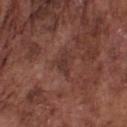The lesion was tiled from a total-body skin photograph and was not biopsied. A male patient, aged approximately 75. Located on the chest. Cropped from a whole-body photographic skin survey; the tile spans about 15 mm. Captured under white-light illumination. The total-body-photography lesion software estimated a footprint of about 3 mm², an outline eccentricity of about 0.85 (0 = round, 1 = elongated), and a symmetry-axis asymmetry near 0.5. It also reported roughly 7 lightness units darker than nearby skin and a lesion-to-skin contrast of about 6 (normalized; higher = more distinct). And it measured a nevus-likeness score of about 0/100 and lesion-presence confidence of about 95/100. The lesion's longest dimension is about 2.5 mm.